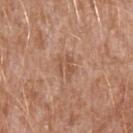<record>
<biopsy_status>not biopsied; imaged during a skin examination</biopsy_status>
<automated_metrics>
  <cielab_L>53</cielab_L>
  <cielab_a>21</cielab_a>
  <cielab_b>31</cielab_b>
  <vs_skin_darker_L>7.0</vs_skin_darker_L>
  <vs_skin_contrast_norm>5.5</vs_skin_contrast_norm>
</automated_metrics>
<site>left upper arm</site>
<patient>
  <sex>male</sex>
  <age_approx>45</age_approx>
</patient>
<lesion_size>
  <long_diameter_mm_approx>3.0</long_diameter_mm_approx>
</lesion_size>
<image>
  <source>total-body photography crop</source>
  <field_of_view_mm>15</field_of_view_mm>
</image>
</record>